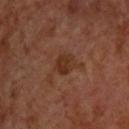tile lighting = cross-polarized
location = the back
automated metrics = a lesion color around L≈30 a*≈20 b*≈28 in CIELAB and roughly 7 lightness units darker than nearby skin; a border-irregularity rating of about 1.5/10, a color-variation rating of about 3/10, and a peripheral color-asymmetry measure near 1
lesion size = ≈3 mm
subject = male, aged approximately 70
acquisition = 15 mm crop, total-body photography